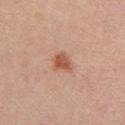Assessment: Recorded during total-body skin imaging; not selected for excision or biopsy. Context: Captured under white-light illumination. The lesion is located on the back. A roughly 15 mm field-of-view crop from a total-body skin photograph. A female patient aged around 25. Longest diameter approximately 2.5 mm.